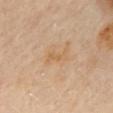workup = imaged on a skin check; not biopsied
illumination = cross-polarized
automated lesion analysis = an area of roughly 3.5 mm² and a shape eccentricity near 0.85
image source = total-body-photography crop, ~15 mm field of view
patient = female, approximately 60 years of age
anatomic site = the back
lesion size = ≈3 mm